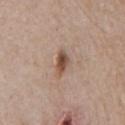The lesion was tiled from a total-body skin photograph and was not biopsied. A male patient, in their 80s. Located on the chest. A lesion tile, about 15 mm wide, cut from a 3D total-body photograph. The lesion's longest dimension is about 3 mm. This is a white-light tile.